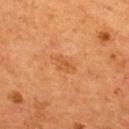workup: imaged on a skin check; not biopsied
anatomic site: the upper back
lesion size: about 3 mm
imaging modality: ~15 mm tile from a whole-body skin photo
image-analysis metrics: an eccentricity of roughly 0.8 and a shape-asymmetry score of about 0.35 (0 = symmetric); a lesion color around L≈52 a*≈26 b*≈41 in CIELAB and about 6 CIELAB-L* units darker than the surrounding skin; a classifier nevus-likeness of about 0/100 and a lesion-detection confidence of about 100/100
patient: male, aged around 55
lighting: cross-polarized illumination